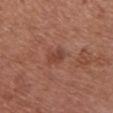This lesion was catalogued during total-body skin photography and was not selected for biopsy.
About 2.5 mm across.
Cropped from a whole-body photographic skin survey; the tile spans about 15 mm.
Located on the chest.
A male patient, in their mid-70s.
Imaged with white-light lighting.